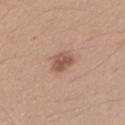No biopsy was performed on this lesion — it was imaged during a full skin examination and was not determined to be concerning.